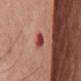workup: total-body-photography surveillance lesion; no biopsy | anatomic site: the chest | imaging modality: ~15 mm tile from a whole-body skin photo | subject: male, aged approximately 75.